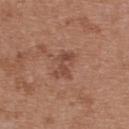Context:
An algorithmic analysis of the crop reported an area of roughly 5 mm² and two-axis asymmetry of about 0.35. Captured under white-light illumination. A lesion tile, about 15 mm wide, cut from a 3D total-body photograph. From the upper back. A female subject, about 40 years old. The lesion's longest dimension is about 3.5 mm.